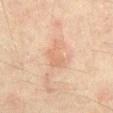{
  "biopsy_status": "not biopsied; imaged during a skin examination",
  "patient": {
    "sex": "male",
    "age_approx": 45
  },
  "site": "abdomen",
  "lighting": "cross-polarized",
  "lesion_size": {
    "long_diameter_mm_approx": 3.5
  },
  "image": {
    "source": "total-body photography crop",
    "field_of_view_mm": 15
  }
}